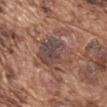Q: Was a biopsy performed?
A: total-body-photography surveillance lesion; no biopsy
Q: What kind of image is this?
A: ~15 mm crop, total-body skin-cancer survey
Q: How was the tile lit?
A: white-light illumination
Q: How large is the lesion?
A: ~6 mm (longest diameter)
Q: What is the anatomic site?
A: the left upper arm
Q: Who is the patient?
A: male, in their mid-70s